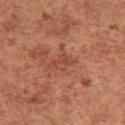Q: What is the anatomic site?
A: the left upper arm
Q: Patient demographics?
A: female, aged 48 to 52
Q: What lighting was used for the tile?
A: white-light illumination
Q: How was this image acquired?
A: ~15 mm tile from a whole-body skin photo
Q: What did automated image analysis measure?
A: an area of roughly 4 mm², an eccentricity of roughly 0.85, and two-axis asymmetry of about 0.3; an average lesion color of about L≈48 a*≈27 b*≈30 (CIELAB), about 7 CIELAB-L* units darker than the surrounding skin, and a lesion-to-skin contrast of about 5 (normalized; higher = more distinct); a nevus-likeness score of about 0/100 and lesion-presence confidence of about 100/100